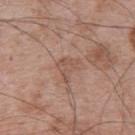The lesion-visualizer software estimated an area of roughly 5 mm² and a symmetry-axis asymmetry near 0.5. And it measured a mean CIELAB color near L≈53 a*≈19 b*≈26, about 7 CIELAB-L* units darker than the surrounding skin, and a normalized border contrast of about 5. And it measured a border-irregularity index near 5/10 and radial color variation of about 0.5. The software also gave a nevus-likeness score of about 0/100 and a lesion-detection confidence of about 95/100.
The tile uses white-light illumination.
A male subject approximately 65 years of age.
Located on the upper back.
Measured at roughly 3 mm in maximum diameter.
A region of skin cropped from a whole-body photographic capture, roughly 15 mm wide.